A male patient about 85 years old or older. Imaged with cross-polarized lighting. A lesion tile, about 15 mm wide, cut from a 3D total-body photograph. The lesion-visualizer software estimated a lesion color around L≈50 a*≈26 b*≈32 in CIELAB, roughly 4 lightness units darker than nearby skin, and a normalized border contrast of about 3.5. And it measured a border-irregularity rating of about 3.5/10 and radial color variation of about 0. It also reported a classifier nevus-likeness of about 0/100. About 2 mm across. On the chest:
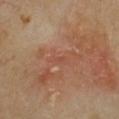Conclusion: Histopathological examination showed a squamous cell carcinoma in situ.